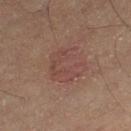Captured during whole-body skin photography for melanoma surveillance; the lesion was not biopsied. The lesion is located on the leg. The patient is a male aged approximately 55. Cropped from a whole-body photographic skin survey; the tile spans about 15 mm. The tile uses cross-polarized illumination. The recorded lesion diameter is about 4.5 mm.